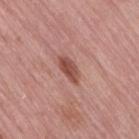{
  "biopsy_status": "not biopsied; imaged during a skin examination",
  "image": {
    "source": "total-body photography crop",
    "field_of_view_mm": 15
  },
  "site": "right thigh",
  "patient": {
    "sex": "female",
    "age_approx": 65
  },
  "lighting": "white-light",
  "automated_metrics": {
    "cielab_L": 50,
    "cielab_a": 25,
    "cielab_b": 26,
    "vs_skin_darker_L": 12.0,
    "vs_skin_contrast_norm": 8.5,
    "border_irregularity_0_10": 2.5,
    "color_variation_0_10": 4.0,
    "peripheral_color_asymmetry": 1.5,
    "lesion_detection_confidence_0_100": 100
  }
}